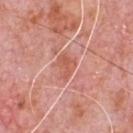notes=total-body-photography surveillance lesion; no biopsy
patient=male, about 80 years old
image=15 mm crop, total-body photography
anatomic site=the chest
lighting=white-light illumination